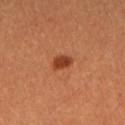Part of a total-body skin-imaging series; this lesion was reviewed on a skin check and was not flagged for biopsy.